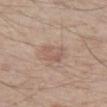The lesion was photographed on a routine skin check and not biopsied; there is no pathology result. The lesion-visualizer software estimated a footprint of about 6.5 mm², a shape eccentricity near 0.4, and a shape-asymmetry score of about 0.25 (0 = symmetric). It also reported an average lesion color of about L≈57 a*≈18 b*≈26 (CIELAB) and a lesion-to-skin contrast of about 5 (normalized; higher = more distinct). The software also gave a border-irregularity rating of about 2.5/10 and a peripheral color-asymmetry measure near 1. The analysis additionally found an automated nevus-likeness rating near 10 out of 100 and a lesion-detection confidence of about 100/100. A male subject, approximately 65 years of age. The tile uses white-light illumination. A lesion tile, about 15 mm wide, cut from a 3D total-body photograph. On the left thigh. The recorded lesion diameter is about 3 mm.